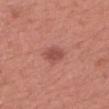Findings:
– follow-up — catalogued during a skin exam; not biopsied
– site — the head or neck
– patient — female, in their mid-60s
– diameter — about 2.5 mm
– image-analysis metrics — an average lesion color of about L≈50 a*≈28 b*≈27 (CIELAB) and a normalized border contrast of about 7; a border-irregularity index near 2/10, a color-variation rating of about 2/10, and radial color variation of about 1; a classifier nevus-likeness of about 80/100 and a lesion-detection confidence of about 100/100
– imaging modality — ~15 mm tile from a whole-body skin photo
– lighting — white-light